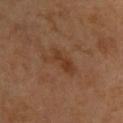No biopsy was performed on this lesion — it was imaged during a full skin examination and was not determined to be concerning. A 15 mm crop from a total-body photograph taken for skin-cancer surveillance. The subject is a male aged approximately 45. From the upper back.